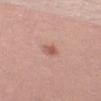Recorded during total-body skin imaging; not selected for excision or biopsy. A female patient approximately 40 years of age. Located on the left thigh. Longest diameter approximately 2.5 mm. An algorithmic analysis of the crop reported an area of roughly 3 mm² and an outline eccentricity of about 0.8 (0 = round, 1 = elongated). The analysis additionally found an average lesion color of about L≈57 a*≈24 b*≈27 (CIELAB), a lesion–skin lightness drop of about 10, and a normalized lesion–skin contrast near 6.5. Captured under white-light illumination. A region of skin cropped from a whole-body photographic capture, roughly 15 mm wide.